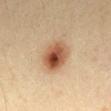Captured during whole-body skin photography for melanoma surveillance; the lesion was not biopsied.
The tile uses cross-polarized illumination.
The lesion is located on the back.
A male patient aged approximately 35.
A lesion tile, about 15 mm wide, cut from a 3D total-body photograph.
The total-body-photography lesion software estimated a lesion color around L≈47 a*≈20 b*≈31 in CIELAB, roughly 15 lightness units darker than nearby skin, and a lesion-to-skin contrast of about 10.5 (normalized; higher = more distinct).
The recorded lesion diameter is about 4 mm.